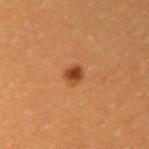{"biopsy_status": "not biopsied; imaged during a skin examination"}